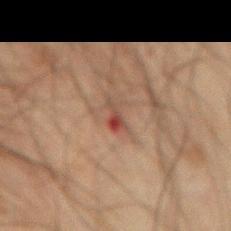Assessment: No biopsy was performed on this lesion — it was imaged during a full skin examination and was not determined to be concerning.